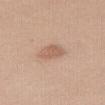Impression: This lesion was catalogued during total-body skin photography and was not selected for biopsy. Image and clinical context: A female subject in their 40s. Cropped from a total-body skin-imaging series; the visible field is about 15 mm. The lesion-visualizer software estimated a mean CIELAB color near L≈60 a*≈19 b*≈29 and a lesion-to-skin contrast of about 6 (normalized; higher = more distinct). It also reported a border-irregularity index near 2.5/10, a color-variation rating of about 2.5/10, and a peripheral color-asymmetry measure near 1. It also reported a classifier nevus-likeness of about 40/100 and lesion-presence confidence of about 100/100. From the right thigh. The tile uses white-light illumination. Longest diameter approximately 3 mm.